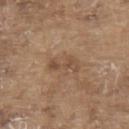{"image": {"source": "total-body photography crop", "field_of_view_mm": 15}, "lesion_size": {"long_diameter_mm_approx": 4.0}, "lighting": "white-light", "patient": {"sex": "male", "age_approx": 80}, "site": "back", "automated_metrics": {"area_mm2_approx": 5.0, "eccentricity": 0.95, "shape_asymmetry": 0.45}}